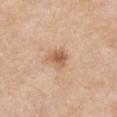Part of a total-body skin-imaging series; this lesion was reviewed on a skin check and was not flagged for biopsy. Imaged with white-light lighting. Approximately 2.5 mm at its widest. The patient is a male aged 78 to 82. The lesion is on the right upper arm. A 15 mm close-up tile from a total-body photography series done for melanoma screening.